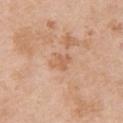Captured during whole-body skin photography for melanoma surveillance; the lesion was not biopsied. The lesion is on the front of the torso. A region of skin cropped from a whole-body photographic capture, roughly 15 mm wide. A female subject aged approximately 40.